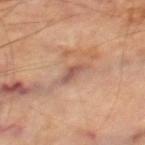The lesion was photographed on a routine skin check and not biopsied; there is no pathology result.